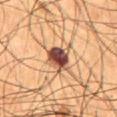<case>
<biopsy_status>not biopsied; imaged during a skin examination</biopsy_status>
<lesion_size>
  <long_diameter_mm_approx>4.0</long_diameter_mm_approx>
</lesion_size>
<site>abdomen</site>
<image>
  <source>total-body photography crop</source>
  <field_of_view_mm>15</field_of_view_mm>
</image>
<patient>
  <sex>male</sex>
  <age_approx>55</age_approx>
</patient>
</case>